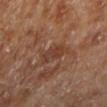notes = no biopsy performed (imaged during a skin exam)
image source = 15 mm crop, total-body photography
tile lighting = cross-polarized
location = the left lower leg
subject = male, aged 68 to 72
TBP lesion metrics = border irregularity of about 3 on a 0–10 scale, a color-variation rating of about 3.5/10, and radial color variation of about 1; lesion-presence confidence of about 100/100